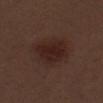Automated image analysis of the tile measured a mean CIELAB color near L≈22 a*≈18 b*≈19 and a lesion-to-skin contrast of about 8 (normalized; higher = more distinct). The software also gave border irregularity of about 2.5 on a 0–10 scale, internal color variation of about 2.5 on a 0–10 scale, and peripheral color asymmetry of about 1. The software also gave a nevus-likeness score of about 90/100. Measured at roughly 4.5 mm in maximum diameter. Imaged with white-light lighting. Cropped from a total-body skin-imaging series; the visible field is about 15 mm. On the left thigh. A male patient approximately 70 years of age.